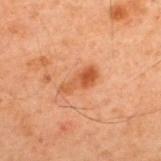biopsy status: catalogued during a skin exam; not biopsied
size: ~4 mm (longest diameter)
acquisition: total-body-photography crop, ~15 mm field of view
site: the upper back
subject: male, about 60 years old
tile lighting: cross-polarized illumination